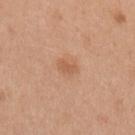biopsy_status: not biopsied; imaged during a skin examination
image:
  source: total-body photography crop
  field_of_view_mm: 15
patient:
  sex: female
  age_approx: 40
site: left upper arm